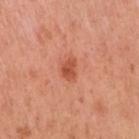Automated tile analysis of the lesion measured a lesion area of about 4 mm², an eccentricity of roughly 0.7, and a shape-asymmetry score of about 0.25 (0 = symmetric). The analysis additionally found a lesion color around L≈54 a*≈32 b*≈36 in CIELAB, a lesion–skin lightness drop of about 11, and a normalized lesion–skin contrast near 7.5. The analysis additionally found a border-irregularity rating of about 2.5/10. It also reported a classifier nevus-likeness of about 95/100 and a detector confidence of about 100 out of 100 that the crop contains a lesion.
Imaged with white-light lighting.
A female subject, about 50 years old.
About 2.5 mm across.
Cropped from a whole-body photographic skin survey; the tile spans about 15 mm.
The lesion is located on the right upper arm.